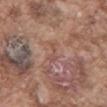No biopsy was performed on this lesion — it was imaged during a full skin examination and was not determined to be concerning.
A close-up tile cropped from a whole-body skin photograph, about 15 mm across.
From the abdomen.
A male subject, aged approximately 75.